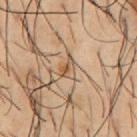notes: no biopsy performed (imaged during a skin exam) | acquisition: ~15 mm tile from a whole-body skin photo | image-analysis metrics: a footprint of about 3.5 mm² and a shape-asymmetry score of about 0.5 (0 = symmetric); an average lesion color of about L≈54 a*≈17 b*≈32 (CIELAB), roughly 10 lightness units darker than nearby skin, and a lesion-to-skin contrast of about 7 (normalized; higher = more distinct); lesion-presence confidence of about 70/100 | lighting: cross-polarized | patient: male, aged 53 to 57 | site: the chest.